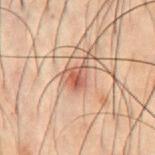Q: Is there a histopathology result?
A: no biopsy performed (imaged during a skin exam)
Q: What kind of image is this?
A: total-body-photography crop, ~15 mm field of view
Q: What are the patient's age and sex?
A: male, in their 50s
Q: Lesion size?
A: ~3 mm (longest diameter)
Q: Lesion location?
A: the chest
Q: Illumination type?
A: cross-polarized